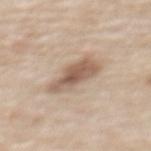Q: Was a biopsy performed?
A: imaged on a skin check; not biopsied
Q: How was this image acquired?
A: ~15 mm tile from a whole-body skin photo
Q: What did automated image analysis measure?
A: an automated nevus-likeness rating near 25 out of 100 and a lesion-detection confidence of about 100/100
Q: How was the tile lit?
A: white-light illumination
Q: Who is the patient?
A: female, aged 63 to 67
Q: Where on the body is the lesion?
A: the back